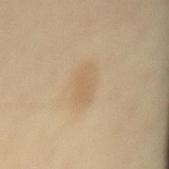Q: Was this lesion biopsied?
A: no biopsy performed (imaged during a skin exam)
Q: Patient demographics?
A: female, aged 43 to 47
Q: What is the anatomic site?
A: the mid back
Q: What did automated image analysis measure?
A: a mean CIELAB color near L≈63 a*≈13 b*≈34 and roughly 6 lightness units darker than nearby skin; border irregularity of about 2.5 on a 0–10 scale, a color-variation rating of about 1.5/10, and radial color variation of about 0.5
Q: What is the imaging modality?
A: ~15 mm crop, total-body skin-cancer survey